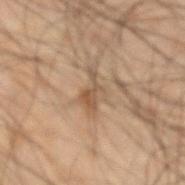Imaged during a routine full-body skin examination; the lesion was not biopsied and no histopathology is available. The patient is a male approximately 50 years of age. Captured under cross-polarized illumination. This image is a 15 mm lesion crop taken from a total-body photograph. The lesion's longest dimension is about 4.5 mm. Located on the arm.